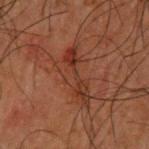| feature | finding |
|---|---|
| notes | catalogued during a skin exam; not biopsied |
| patient | male, approximately 50 years of age |
| imaging modality | ~15 mm crop, total-body skin-cancer survey |
| body site | the back |
| diameter | about 6.5 mm |